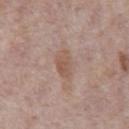Q: Was this lesion biopsied?
A: no biopsy performed (imaged during a skin exam)
Q: What are the patient's age and sex?
A: male, aged 68 to 72
Q: Lesion size?
A: about 3.5 mm
Q: How was this image acquired?
A: ~15 mm crop, total-body skin-cancer survey
Q: How was the tile lit?
A: white-light illumination
Q: Where on the body is the lesion?
A: the abdomen